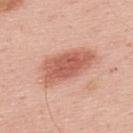The lesion was photographed on a routine skin check and not biopsied; there is no pathology result.
A 15 mm close-up extracted from a 3D total-body photography capture.
A male patient, about 65 years old.
Imaged with white-light lighting.
Longest diameter approximately 6.5 mm.
From the back.